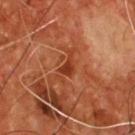Case summary:
* workup — catalogued during a skin exam; not biopsied
* anatomic site — the chest
* imaging modality — ~15 mm crop, total-body skin-cancer survey
* patient — male, aged 53 to 57
* lesion diameter — about 2.5 mm
* tile lighting — cross-polarized illumination